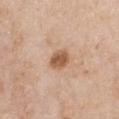notes: total-body-photography surveillance lesion; no biopsy | body site: the chest | acquisition: ~15 mm crop, total-body skin-cancer survey | subject: female, in their 70s.